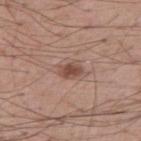notes — catalogued during a skin exam; not biopsied
lesion diameter — ~2.5 mm (longest diameter)
automated metrics — a lesion area of about 4 mm², an eccentricity of roughly 0.75, and a symmetry-axis asymmetry near 0.25; internal color variation of about 2 on a 0–10 scale; an automated nevus-likeness rating near 90 out of 100 and lesion-presence confidence of about 100/100
patient — male, aged 53–57
acquisition — 15 mm crop, total-body photography
illumination — white-light